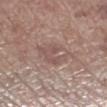The lesion was photographed on a routine skin check and not biopsied; there is no pathology result. The total-body-photography lesion software estimated a border-irregularity index near 3/10 and peripheral color asymmetry of about 2. On the left lower leg. This image is a 15 mm lesion crop taken from a total-body photograph. The recorded lesion diameter is about 5 mm. The patient is a male aged 68–72.